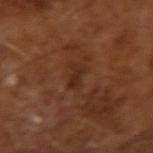{
  "biopsy_status": "not biopsied; imaged during a skin examination",
  "image": {
    "source": "total-body photography crop",
    "field_of_view_mm": 15
  },
  "patient": {
    "sex": "male",
    "age_approx": 65
  }
}